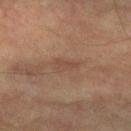Recorded during total-body skin imaging; not selected for excision or biopsy. The lesion is located on the arm. About 3.5 mm across. A close-up tile cropped from a whole-body skin photograph, about 15 mm across. The patient is a male aged 73–77.